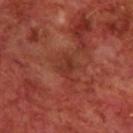The lesion was tiled from a total-body skin photograph and was not biopsied.
Cropped from a whole-body photographic skin survey; the tile spans about 15 mm.
The lesion is located on the upper back.
A male patient, in their 70s.